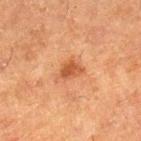No biopsy was performed on this lesion — it was imaged during a full skin examination and was not determined to be concerning.
The lesion is located on the right lower leg.
About 2.5 mm across.
The tile uses cross-polarized illumination.
A female patient, about 50 years old.
A 15 mm close-up extracted from a 3D total-body photography capture.